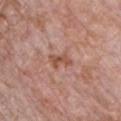tile lighting = white-light illumination
patient = male, roughly 70 years of age
location = the front of the torso
diameter = ≈3 mm
image source = total-body-photography crop, ~15 mm field of view
TBP lesion metrics = a footprint of about 4 mm², an eccentricity of roughly 0.85, and a symmetry-axis asymmetry near 0.3; a lesion color around L≈52 a*≈23 b*≈29 in CIELAB and roughly 9 lightness units darker than nearby skin; a border-irregularity rating of about 3.5/10